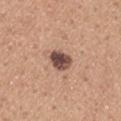This lesion was catalogued during total-body skin photography and was not selected for biopsy.
The subject is a male roughly 45 years of age.
A 15 mm crop from a total-body photograph taken for skin-cancer surveillance.
An algorithmic analysis of the crop reported a lesion–skin lightness drop of about 18 and a normalized border contrast of about 13. And it measured a border-irregularity index near 1.5/10, internal color variation of about 5.5 on a 0–10 scale, and radial color variation of about 1.5. And it measured a classifier nevus-likeness of about 30/100 and lesion-presence confidence of about 100/100.
This is a white-light tile.
Measured at roughly 3 mm in maximum diameter.
On the right upper arm.